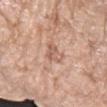Impression:
Part of a total-body skin-imaging series; this lesion was reviewed on a skin check and was not flagged for biopsy.
Background:
A region of skin cropped from a whole-body photographic capture, roughly 15 mm wide. On the right forearm. A female patient, about 75 years old.